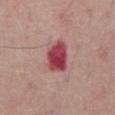| key | value |
|---|---|
| biopsy status | imaged on a skin check; not biopsied |